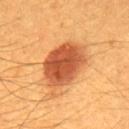This lesion was catalogued during total-body skin photography and was not selected for biopsy.
Measured at roughly 6.5 mm in maximum diameter.
The patient is a male about 60 years old.
Located on the back.
Captured under cross-polarized illumination.
Automated tile analysis of the lesion measured lesion-presence confidence of about 100/100.
This image is a 15 mm lesion crop taken from a total-body photograph.